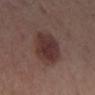The lesion was photographed on a routine skin check and not biopsied; there is no pathology result.
An algorithmic analysis of the crop reported a footprint of about 18 mm², an outline eccentricity of about 0.6 (0 = round, 1 = elongated), and two-axis asymmetry of about 0.1. And it measured a nevus-likeness score of about 80/100 and a detector confidence of about 100 out of 100 that the crop contains a lesion.
The lesion is on the right lower leg.
Imaged with white-light lighting.
About 5.5 mm across.
A close-up tile cropped from a whole-body skin photograph, about 15 mm across.
The patient is a female about 40 years old.